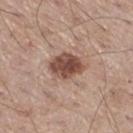{
  "biopsy_status": "not biopsied; imaged during a skin examination",
  "patient": {
    "sex": "male",
    "age_approx": 60
  },
  "site": "leg",
  "automated_metrics": {
    "area_mm2_approx": 9.5,
    "cielab_L": 48,
    "cielab_a": 20,
    "cielab_b": 25,
    "vs_skin_darker_L": 16.0,
    "vs_skin_contrast_norm": 11.0,
    "border_irregularity_0_10": 2.0,
    "color_variation_0_10": 4.5,
    "peripheral_color_asymmetry": 1.5,
    "nevus_likeness_0_100": 90
  },
  "image": {
    "source": "total-body photography crop",
    "field_of_view_mm": 15
  },
  "lesion_size": {
    "long_diameter_mm_approx": 4.0
  }
}